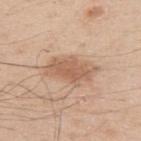Clinical impression:
The lesion was photographed on a routine skin check and not biopsied; there is no pathology result.
Image and clinical context:
The lesion is on the arm. This is a white-light tile. Cropped from a total-body skin-imaging series; the visible field is about 15 mm. The lesion's longest dimension is about 5.5 mm. A male patient about 30 years old.